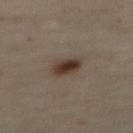Context:
Automated tile analysis of the lesion measured a mean CIELAB color near L≈36 a*≈13 b*≈23 and a lesion–skin lightness drop of about 11. It also reported radial color variation of about 1.5. A close-up tile cropped from a whole-body skin photograph, about 15 mm across. From the abdomen. Approximately 3 mm at its widest. Imaged with cross-polarized lighting. A female subject, aged 58–62.